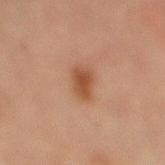location: the right lower leg | acquisition: ~15 mm crop, total-body skin-cancer survey | patient: female, aged around 70.